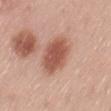* follow-up: imaged on a skin check; not biopsied
* TBP lesion metrics: a lesion area of about 15 mm² and a symmetry-axis asymmetry near 0.15; a lesion color around L≈55 a*≈24 b*≈29 in CIELAB, about 13 CIELAB-L* units darker than the surrounding skin, and a normalized lesion–skin contrast near 9; a border-irregularity rating of about 1.5/10, a color-variation rating of about 3.5/10, and a peripheral color-asymmetry measure near 1
* diameter: about 5.5 mm
* lighting: white-light illumination
* subject: male, aged 48 to 52
* site: the back
* imaging modality: total-body-photography crop, ~15 mm field of view